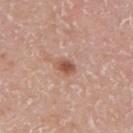<lesion>
<biopsy_status>not biopsied; imaged during a skin examination</biopsy_status>
<automated_metrics>
  <nevus_likeness_0_100>95</nevus_likeness_0_100>
  <lesion_detection_confidence_0_100>100</lesion_detection_confidence_0_100>
</automated_metrics>
<patient>
  <sex>female</sex>
  <age_approx>30</age_approx>
</patient>
<image>
  <source>total-body photography crop</source>
  <field_of_view_mm>15</field_of_view_mm>
</image>
<site>back</site>
</lesion>